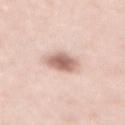{"biopsy_status": "not biopsied; imaged during a skin examination", "patient": {"sex": "female", "age_approx": 40}, "automated_metrics": {"area_mm2_approx": 7.5, "eccentricity": 0.65, "shape_asymmetry": 0.2, "nevus_likeness_0_100": 90}, "image": {"source": "total-body photography crop", "field_of_view_mm": 15}, "lesion_size": {"long_diameter_mm_approx": 3.5}, "site": "mid back"}